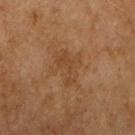Image and clinical context:
Located on the right upper arm. Captured under cross-polarized illumination. A female patient, aged around 60. A lesion tile, about 15 mm wide, cut from a 3D total-body photograph.